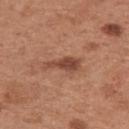Impression: No biopsy was performed on this lesion — it was imaged during a full skin examination and was not determined to be concerning. Background: Located on the left upper arm. About 4.5 mm across. The tile uses white-light illumination. A female patient, aged 28–32. A 15 mm close-up tile from a total-body photography series done for melanoma screening.